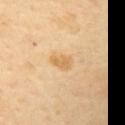Impression: The lesion was photographed on a routine skin check and not biopsied; there is no pathology result. Clinical summary: Cropped from a whole-body photographic skin survey; the tile spans about 15 mm. A female subject, aged approximately 60. The tile uses cross-polarized illumination. Automated tile analysis of the lesion measured a lesion area of about 4 mm², a shape eccentricity near 0.8, and two-axis asymmetry of about 0.25. The analysis additionally found a lesion color around L≈70 a*≈18 b*≈45 in CIELAB, a lesion–skin lightness drop of about 8, and a normalized lesion–skin contrast near 6.5. And it measured border irregularity of about 2.5 on a 0–10 scale, a color-variation rating of about 2/10, and radial color variation of about 0.5. Longest diameter approximately 2.5 mm. The lesion is located on the mid back.